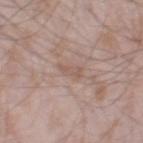Captured during whole-body skin photography for melanoma surveillance; the lesion was not biopsied. This image is a 15 mm lesion crop taken from a total-body photograph. A male patient, aged approximately 45. The lesion is located on the right thigh.